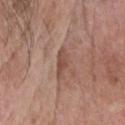The lesion was tiled from a total-body skin photograph and was not biopsied. From the head or neck. The lesion-visualizer software estimated a lesion color around L≈49 a*≈20 b*≈26 in CIELAB and a lesion-to-skin contrast of about 7 (normalized; higher = more distinct). The software also gave a border-irregularity rating of about 3.5/10, a within-lesion color-variation index near 2/10, and radial color variation of about 0.5. The tile uses white-light illumination. The lesion's longest dimension is about 3.5 mm. A male subject, aged around 75. A close-up tile cropped from a whole-body skin photograph, about 15 mm across.